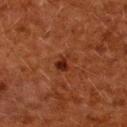• workup — no biopsy performed (imaged during a skin exam)
• anatomic site — the upper back
• lesion diameter — ≈2.5 mm
• acquisition — ~15 mm tile from a whole-body skin photo
• patient — female, aged around 50
• illumination — cross-polarized illumination